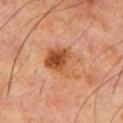Imaged during a routine full-body skin examination; the lesion was not biopsied and no histopathology is available.
A region of skin cropped from a whole-body photographic capture, roughly 15 mm wide.
This is a cross-polarized tile.
The lesion is on the chest.
The recorded lesion diameter is about 5 mm.
The patient is a male aged 68 to 72.